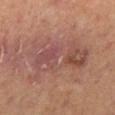workup — no biopsy performed (imaged during a skin exam) | lesion diameter — ≈9 mm | image — ~15 mm tile from a whole-body skin photo | tile lighting — cross-polarized | subject — male, about 65 years old | body site — the left lower leg.